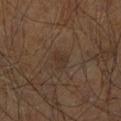Clinical impression: This lesion was catalogued during total-body skin photography and was not selected for biopsy. Image and clinical context: Captured under cross-polarized illumination. Cropped from a whole-body photographic skin survey; the tile spans about 15 mm. Longest diameter approximately 3 mm. The lesion is located on the right leg. A male subject, about 60 years old.